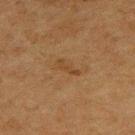biopsy status — imaged on a skin check; not biopsied
diameter — ≈3 mm
image — total-body-photography crop, ~15 mm field of view
location — the upper back
tile lighting — cross-polarized illumination
subject — male, aged 73–77